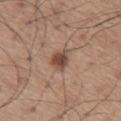This lesion was catalogued during total-body skin photography and was not selected for biopsy. The recorded lesion diameter is about 3 mm. Located on the left thigh. The patient is a male aged 63–67. This image is a 15 mm lesion crop taken from a total-body photograph. Imaged with white-light lighting.